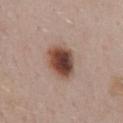The lesion was photographed on a routine skin check and not biopsied; there is no pathology result.
The subject is a male approximately 55 years of age.
Captured under white-light illumination.
The lesion is on the mid back.
Cropped from a whole-body photographic skin survey; the tile spans about 15 mm.